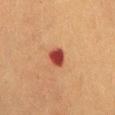acquisition — total-body-photography crop, ~15 mm field of view | subject — female, aged approximately 50 | body site — the abdomen | automated lesion analysis — an area of roughly 4.5 mm², an eccentricity of roughly 0.5, and a shape-asymmetry score of about 0.2 (0 = symmetric); roughly 15 lightness units darker than nearby skin and a normalized lesion–skin contrast near 12.5; a color-variation rating of about 2/10; a classifier nevus-likeness of about 0/100 and lesion-presence confidence of about 100/100 | size — about 2.5 mm.